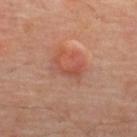Notes:
- workup · imaged on a skin check; not biopsied
- tile lighting · cross-polarized
- image · 15 mm crop, total-body photography
- size · about 3.5 mm
- subject · male, aged 63–67
- location · the upper back